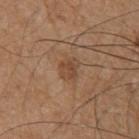Acquisition and patient details: The total-body-photography lesion software estimated a lesion area of about 4.5 mm² and an outline eccentricity of about 0.5 (0 = round, 1 = elongated). The software also gave a mean CIELAB color near L≈46 a*≈19 b*≈30, roughly 8 lightness units darker than nearby skin, and a normalized lesion–skin contrast near 6. The software also gave border irregularity of about 1.5 on a 0–10 scale, a color-variation rating of about 2.5/10, and a peripheral color-asymmetry measure near 1. The software also gave an automated nevus-likeness rating near 25 out of 100 and a lesion-detection confidence of about 100/100. A lesion tile, about 15 mm wide, cut from a 3D total-body photograph. The subject is a male aged 68 to 72. On the chest. The lesion's longest dimension is about 2.5 mm.